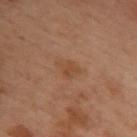Impression:
Recorded during total-body skin imaging; not selected for excision or biopsy.
Clinical summary:
A female patient aged 53–57. Cropped from a total-body skin-imaging series; the visible field is about 15 mm. The tile uses cross-polarized illumination. On the upper back. The total-body-photography lesion software estimated a lesion area of about 4 mm², a shape eccentricity near 0.75, and two-axis asymmetry of about 0.45. And it measured a border-irregularity rating of about 4/10, a within-lesion color-variation index near 1.5/10, and radial color variation of about 0.5. The analysis additionally found a nevus-likeness score of about 0/100. The lesion's longest dimension is about 3 mm.